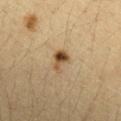– workup — total-body-photography surveillance lesion; no biopsy
– tile lighting — cross-polarized
– image source — ~15 mm tile from a whole-body skin photo
– patient — female, in their 30s
– size — ~3 mm (longest diameter)
– anatomic site — the arm